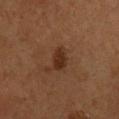Findings:
* biopsy status — total-body-photography surveillance lesion; no biopsy
* acquisition — ~15 mm tile from a whole-body skin photo
* body site — the chest
* subject — male, in their mid-50s